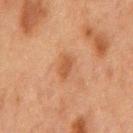Q: Was a biopsy performed?
A: imaged on a skin check; not biopsied
Q: How was this image acquired?
A: ~15 mm tile from a whole-body skin photo
Q: How large is the lesion?
A: ≈2.5 mm
Q: What are the patient's age and sex?
A: male, in their mid- to late 70s
Q: How was the tile lit?
A: cross-polarized
Q: What is the anatomic site?
A: the mid back